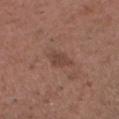Recorded during total-body skin imaging; not selected for excision or biopsy.
Captured under white-light illumination.
The lesion is located on the head or neck.
Measured at roughly 4 mm in maximum diameter.
This image is a 15 mm lesion crop taken from a total-body photograph.
A male patient aged approximately 35.